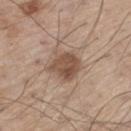The lesion was photographed on a routine skin check and not biopsied; there is no pathology result.
The subject is a male approximately 60 years of age.
A 15 mm close-up extracted from a 3D total-body photography capture.
From the left thigh.
Measured at roughly 4.5 mm in maximum diameter.